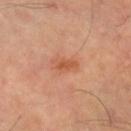The lesion was photographed on a routine skin check and not biopsied; there is no pathology result.
About 3 mm across.
Located on the leg.
This is a cross-polarized tile.
A female subject aged 58–62.
This image is a 15 mm lesion crop taken from a total-body photograph.